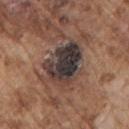Impression: Recorded during total-body skin imaging; not selected for excision or biopsy. Clinical summary: The subject is a male about 75 years old. Longest diameter approximately 5.5 mm. The lesion-visualizer software estimated an area of roughly 17 mm², a shape eccentricity near 0.7, and a shape-asymmetry score of about 0.25 (0 = symmetric). The software also gave a border-irregularity rating of about 2.5/10 and internal color variation of about 9 on a 0–10 scale. Captured under white-light illumination. A roughly 15 mm field-of-view crop from a total-body skin photograph. On the left upper arm.